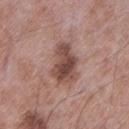The lesion was tiled from a total-body skin photograph and was not biopsied. From the left lower leg. A male patient about 55 years old. A roughly 15 mm field-of-view crop from a total-body skin photograph. Longest diameter approximately 5 mm.